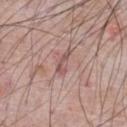A male patient in their 60s. A 15 mm crop from a total-body photograph taken for skin-cancer surveillance. Located on the chest.